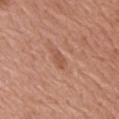Assessment: No biopsy was performed on this lesion — it was imaged during a full skin examination and was not determined to be concerning. Acquisition and patient details: The lesion's longest dimension is about 2.5 mm. Automated tile analysis of the lesion measured an area of roughly 3 mm², an outline eccentricity of about 0.85 (0 = round, 1 = elongated), and two-axis asymmetry of about 0.35. The software also gave a nevus-likeness score of about 0/100. The patient is a female aged approximately 75. A 15 mm close-up tile from a total-body photography series done for melanoma screening. The lesion is located on the back. This is a white-light tile.